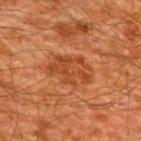lesion size — ~5.5 mm (longest diameter)
subject — male, aged around 60
body site — the upper back
image source — total-body-photography crop, ~15 mm field of view
automated lesion analysis — a footprint of about 14 mm², a shape eccentricity near 0.75, and two-axis asymmetry of about 0.2; an average lesion color of about L≈36 a*≈24 b*≈32 (CIELAB), a lesion–skin lightness drop of about 6, and a lesion-to-skin contrast of about 6 (normalized; higher = more distinct); internal color variation of about 3 on a 0–10 scale
illumination — cross-polarized illumination